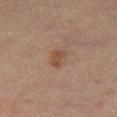Clinical impression:
Captured during whole-body skin photography for melanoma surveillance; the lesion was not biopsied.